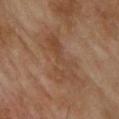This lesion was catalogued during total-body skin photography and was not selected for biopsy. From the upper back. An algorithmic analysis of the crop reported an area of roughly 17 mm² and a symmetry-axis asymmetry near 0.35. The analysis additionally found a mean CIELAB color near L≈44 a*≈18 b*≈30, roughly 6 lightness units darker than nearby skin, and a normalized lesion–skin contrast near 5. It also reported a detector confidence of about 100 out of 100 that the crop contains a lesion. A 15 mm close-up extracted from a 3D total-body photography capture. About 6.5 mm across. A male patient approximately 70 years of age.